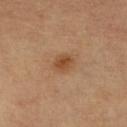Findings:
- notes · catalogued during a skin exam; not biopsied
- imaging modality · ~15 mm crop, total-body skin-cancer survey
- location · the right lower leg
- patient · female, aged 58 to 62